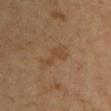The lesion was photographed on a routine skin check and not biopsied; there is no pathology result.
From the chest.
A female patient, roughly 55 years of age.
A lesion tile, about 15 mm wide, cut from a 3D total-body photograph.
This is a cross-polarized tile.